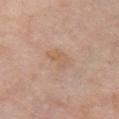Impression: This lesion was catalogued during total-body skin photography and was not selected for biopsy. Context: Automated image analysis of the tile measured a nevus-likeness score of about 0/100 and a lesion-detection confidence of about 100/100. Imaged with white-light lighting. From the front of the torso. A close-up tile cropped from a whole-body skin photograph, about 15 mm across. The subject is a female about 60 years old. Measured at roughly 3.5 mm in maximum diameter.